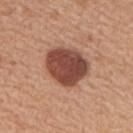Q: What are the patient's age and sex?
A: male, aged 63–67
Q: How large is the lesion?
A: ~5 mm (longest diameter)
Q: What lighting was used for the tile?
A: white-light illumination
Q: What kind of image is this?
A: ~15 mm crop, total-body skin-cancer survey
Q: What is the anatomic site?
A: the upper back